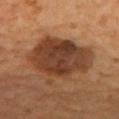Captured during whole-body skin photography for melanoma surveillance; the lesion was not biopsied. The total-body-photography lesion software estimated an area of roughly 33 mm² and an eccentricity of roughly 0.7. It also reported a mean CIELAB color near L≈36 a*≈20 b*≈29, roughly 12 lightness units darker than nearby skin, and a normalized lesion–skin contrast near 10. The analysis additionally found an automated nevus-likeness rating near 70 out of 100 and a lesion-detection confidence of about 100/100. Cropped from a total-body skin-imaging series; the visible field is about 15 mm. Measured at roughly 7.5 mm in maximum diameter. Located on the mid back. Captured under cross-polarized illumination. A male subject, roughly 55 years of age.